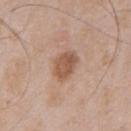– follow-up · no biopsy performed (imaged during a skin exam)
– image · total-body-photography crop, ~15 mm field of view
– illumination · white-light
– lesion diameter · ~3.5 mm (longest diameter)
– body site · the chest
– patient · male, roughly 50 years of age